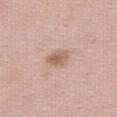A female patient aged approximately 40.
Located on the right thigh.
Cropped from a whole-body photographic skin survey; the tile spans about 15 mm.